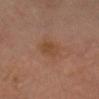• follow-up — no biopsy performed (imaged during a skin exam)
• lighting — cross-polarized illumination
• TBP lesion metrics — a mean CIELAB color near L≈43 a*≈20 b*≈31, a lesion–skin lightness drop of about 6, and a normalized border contrast of about 6; a nevus-likeness score of about 10/100 and lesion-presence confidence of about 100/100
• imaging modality — 15 mm crop, total-body photography
• subject — female, aged around 35
• lesion diameter — ~3 mm (longest diameter)
• location — the head or neck